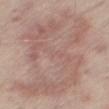Recorded during total-body skin imaging; not selected for excision or biopsy.
Automated tile analysis of the lesion measured a lesion area of about 85 mm² and a shape eccentricity near 0.75. The analysis additionally found a mean CIELAB color near L≈59 a*≈18 b*≈23, about 7 CIELAB-L* units darker than the surrounding skin, and a normalized lesion–skin contrast near 5.
A region of skin cropped from a whole-body photographic capture, roughly 15 mm wide.
Longest diameter approximately 13 mm.
A male subject, about 65 years old.
Captured under white-light illumination.
On the right thigh.